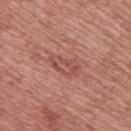A female patient, aged 38 to 42. A 15 mm close-up tile from a total-body photography series done for melanoma screening. The lesion is on the upper back.